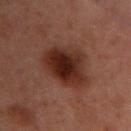| feature | finding |
|---|---|
| notes | imaged on a skin check; not biopsied |
| site | the chest |
| subject | female, aged 53 to 57 |
| acquisition | ~15 mm tile from a whole-body skin photo |